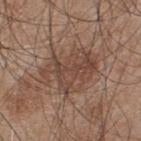Recorded during total-body skin imaging; not selected for excision or biopsy. The lesion-visualizer software estimated a mean CIELAB color near L≈45 a*≈18 b*≈26, about 8 CIELAB-L* units darker than the surrounding skin, and a normalized lesion–skin contrast near 6. And it measured lesion-presence confidence of about 100/100. The tile uses white-light illumination. The subject is a male aged around 45. The lesion is on the upper back. A roughly 15 mm field-of-view crop from a total-body skin photograph. The lesion's longest dimension is about 6 mm.